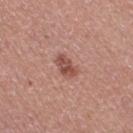Q: Is there a histopathology result?
A: total-body-photography surveillance lesion; no biopsy
Q: What are the patient's age and sex?
A: female, about 50 years old
Q: What kind of image is this?
A: ~15 mm crop, total-body skin-cancer survey
Q: What is the anatomic site?
A: the right thigh
Q: What is the lesion's diameter?
A: ~3 mm (longest diameter)